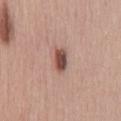{"biopsy_status": "not biopsied; imaged during a skin examination", "automated_metrics": {"area_mm2_approx": 5.0, "eccentricity": 0.85, "shape_asymmetry": 0.25, "border_irregularity_0_10": 2.5, "peripheral_color_asymmetry": 1.5}, "patient": {"sex": "male", "age_approx": 45}, "lesion_size": {"long_diameter_mm_approx": 3.0}, "site": "lower back", "image": {"source": "total-body photography crop", "field_of_view_mm": 15}, "lighting": "white-light"}